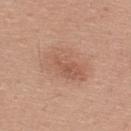Captured under white-light illumination.
A male subject, aged 28 to 32.
Located on the upper back.
A 15 mm close-up tile from a total-body photography series done for melanoma screening.
The recorded lesion diameter is about 6 mm.
An algorithmic analysis of the crop reported about 7 CIELAB-L* units darker than the surrounding skin and a lesion-to-skin contrast of about 5 (normalized; higher = more distinct). The analysis additionally found internal color variation of about 4.5 on a 0–10 scale.